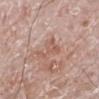follow-up = no biopsy performed (imaged during a skin exam) | anatomic site = the right leg | imaging modality = ~15 mm tile from a whole-body skin photo | lesion size = about 4 mm | patient = male, approximately 70 years of age | illumination = white-light.